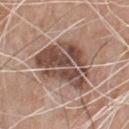follow-up: total-body-photography surveillance lesion; no biopsy | imaging modality: total-body-photography crop, ~15 mm field of view | site: the chest | tile lighting: white-light illumination | patient: male, approximately 80 years of age.